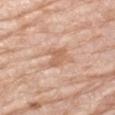{"biopsy_status": "not biopsied; imaged during a skin examination", "patient": {"sex": "female", "age_approx": 75}, "lesion_size": {"long_diameter_mm_approx": 2.5}, "automated_metrics": {"cielab_L": 62, "cielab_a": 21, "cielab_b": 33, "vs_skin_darker_L": 9.0, "border_irregularity_0_10": 3.5, "color_variation_0_10": 1.5, "peripheral_color_asymmetry": 0.5, "nevus_likeness_0_100": 0, "lesion_detection_confidence_0_100": 100}, "image": {"source": "total-body photography crop", "field_of_view_mm": 15}, "site": "left upper arm", "lighting": "white-light"}